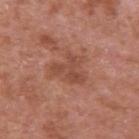Clinical impression: No biopsy was performed on this lesion — it was imaged during a full skin examination and was not determined to be concerning. Acquisition and patient details: This is a white-light tile. Automated image analysis of the tile measured border irregularity of about 8.5 on a 0–10 scale and a within-lesion color-variation index near 2/10. A 15 mm close-up tile from a total-body photography series done for melanoma screening. From the left upper arm. About 4 mm across. A male patient, approximately 65 years of age.